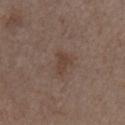Clinical impression: Imaged during a routine full-body skin examination; the lesion was not biopsied and no histopathology is available. Image and clinical context: Cropped from a whole-body photographic skin survey; the tile spans about 15 mm. The lesion's longest dimension is about 2.5 mm. Automated tile analysis of the lesion measured a footprint of about 3.5 mm² and a symmetry-axis asymmetry near 0.45. The analysis additionally found border irregularity of about 4.5 on a 0–10 scale, a within-lesion color-variation index near 1.5/10, and radial color variation of about 0.5. The tile uses white-light illumination. A male patient, aged around 55. On the right upper arm.